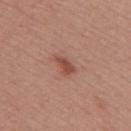Imaged during a routine full-body skin examination; the lesion was not biopsied and no histopathology is available. The lesion is on the back. A female patient about 50 years old. Captured under white-light illumination. Measured at roughly 3 mm in maximum diameter. Automated image analysis of the tile measured a mean CIELAB color near L≈49 a*≈24 b*≈28 and a lesion-to-skin contrast of about 7 (normalized; higher = more distinct). And it measured a border-irregularity rating of about 3.5/10 and a peripheral color-asymmetry measure near 0.5. And it measured a classifier nevus-likeness of about 70/100 and a detector confidence of about 100 out of 100 that the crop contains a lesion. Cropped from a total-body skin-imaging series; the visible field is about 15 mm.